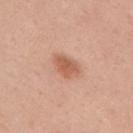Q: Was a biopsy performed?
A: no biopsy performed (imaged during a skin exam)
Q: Who is the patient?
A: female, in their mid-30s
Q: Lesion location?
A: the upper back
Q: What is the lesion's diameter?
A: about 3.5 mm
Q: What is the imaging modality?
A: ~15 mm tile from a whole-body skin photo
Q: Automated lesion metrics?
A: a normalized lesion–skin contrast near 7.5; a border-irregularity rating of about 2.5/10 and internal color variation of about 2 on a 0–10 scale
Q: Illumination type?
A: white-light